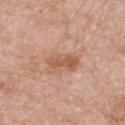follow-up = imaged on a skin check; not biopsied
size = ≈3.5 mm
body site = the right lower leg
subject = female, about 70 years old
illumination = white-light
image = ~15 mm crop, total-body skin-cancer survey